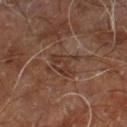The lesion is located on the right leg. The total-body-photography lesion software estimated border irregularity of about 5.5 on a 0–10 scale, a within-lesion color-variation index near 3.5/10, and radial color variation of about 1.5. And it measured a classifier nevus-likeness of about 0/100 and a detector confidence of about 85 out of 100 that the crop contains a lesion. A male patient in their 60s. Captured under cross-polarized illumination. Longest diameter approximately 3.5 mm. A close-up tile cropped from a whole-body skin photograph, about 15 mm across.